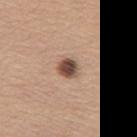Part of a total-body skin-imaging series; this lesion was reviewed on a skin check and was not flagged for biopsy.